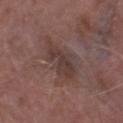No biopsy was performed on this lesion — it was imaged during a full skin examination and was not determined to be concerning. A 15 mm crop from a total-body photograph taken for skin-cancer surveillance. From the right thigh. The subject is a male aged 73–77.